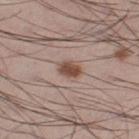Recorded during total-body skin imaging; not selected for excision or biopsy. A male subject, in their mid- to late 30s. This is a white-light tile. A 15 mm crop from a total-body photograph taken for skin-cancer surveillance. The recorded lesion diameter is about 2.5 mm. From the leg.